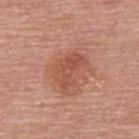{"biopsy_status": "not biopsied; imaged during a skin examination", "patient": {"sex": "male", "age_approx": 80}, "image": {"source": "total-body photography crop", "field_of_view_mm": 15}, "site": "back"}